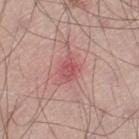follow-up: total-body-photography surveillance lesion; no biopsy | automated lesion analysis: an area of roughly 5 mm², an eccentricity of roughly 0.55, and two-axis asymmetry of about 0.2; a mean CIELAB color near L≈54 a*≈29 b*≈23; a border-irregularity index near 2.5/10, a color-variation rating of about 3/10, and radial color variation of about 1; an automated nevus-likeness rating near 5 out of 100 and a detector confidence of about 100 out of 100 that the crop contains a lesion | location: the left thigh | lighting: white-light illumination | subject: male, roughly 45 years of age | image: total-body-photography crop, ~15 mm field of view | size: ≈2.5 mm.